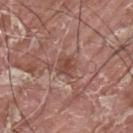Case summary:
* notes: catalogued during a skin exam; not biopsied
* site: the upper back
* subject: male, about 40 years old
* acquisition: total-body-photography crop, ~15 mm field of view
* tile lighting: white-light
* automated lesion analysis: a lesion-detection confidence of about 95/100